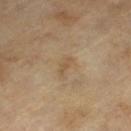No biopsy was performed on this lesion — it was imaged during a full skin examination and was not determined to be concerning.
A roughly 15 mm field-of-view crop from a total-body skin photograph.
Measured at roughly 2.5 mm in maximum diameter.
A male patient in their mid-60s.